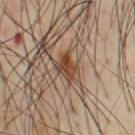This lesion was catalogued during total-body skin photography and was not selected for biopsy. A region of skin cropped from a whole-body photographic capture, roughly 15 mm wide. From the chest. A male subject aged 53–57.